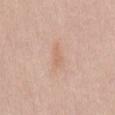This lesion was catalogued during total-body skin photography and was not selected for biopsy. Automated tile analysis of the lesion measured an area of roughly 2.5 mm² and an eccentricity of roughly 0.9. And it measured a border-irregularity index near 4.5/10, a color-variation rating of about 0/10, and radial color variation of about 0. And it measured a classifier nevus-likeness of about 0/100 and a lesion-detection confidence of about 100/100. A female patient aged 38 to 42. Approximately 2.5 mm at its widest. A lesion tile, about 15 mm wide, cut from a 3D total-body photograph. On the back. The tile uses white-light illumination.